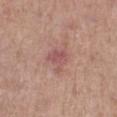This lesion was catalogued during total-body skin photography and was not selected for biopsy.
A 15 mm close-up extracted from a 3D total-body photography capture.
The patient is a female aged around 40.
Automated tile analysis of the lesion measured a footprint of about 5.5 mm², an eccentricity of roughly 0.45, and a symmetry-axis asymmetry near 0.4.
From the right lower leg.
Measured at roughly 3 mm in maximum diameter.
Captured under white-light illumination.